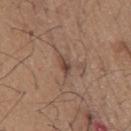This lesion was catalogued during total-body skin photography and was not selected for biopsy. The patient is a male aged around 65. The lesion is located on the front of the torso. The lesion-visualizer software estimated an area of roughly 3 mm² and a symmetry-axis asymmetry near 0.3. The analysis additionally found an automated nevus-likeness rating near 10 out of 100 and lesion-presence confidence of about 100/100. Approximately 2.5 mm at its widest. Cropped from a total-body skin-imaging series; the visible field is about 15 mm.